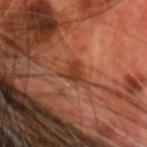<lesion>
<biopsy_status>not biopsied; imaged during a skin examination</biopsy_status>
<patient>
  <sex>male</sex>
  <age_approx>70</age_approx>
</patient>
<lesion_size>
  <long_diameter_mm_approx>3.5</long_diameter_mm_approx>
</lesion_size>
<image>
  <source>total-body photography crop</source>
  <field_of_view_mm>15</field_of_view_mm>
</image>
<site>head or neck</site>
<automated_metrics>
  <eccentricity>0.65</eccentricity>
  <shape_asymmetry>0.55</shape_asymmetry>
  <cielab_L>38</cielab_L>
  <cielab_a>25</cielab_a>
  <cielab_b>32</cielab_b>
  <vs_skin_contrast_norm>7.0</vs_skin_contrast_norm>
  <nevus_likeness_0_100>0</nevus_likeness_0_100>
</automated_metrics>
</lesion>